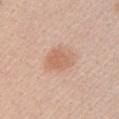Q: Was this lesion biopsied?
A: total-body-photography surveillance lesion; no biopsy
Q: Lesion location?
A: the chest
Q: Lesion size?
A: about 4 mm
Q: What is the imaging modality?
A: ~15 mm tile from a whole-body skin photo
Q: How was the tile lit?
A: white-light illumination
Q: Patient demographics?
A: female, approximately 40 years of age
Q: What did automated image analysis measure?
A: a mean CIELAB color near L≈63 a*≈21 b*≈32 and a lesion-to-skin contrast of about 6 (normalized; higher = more distinct); border irregularity of about 2 on a 0–10 scale, a color-variation rating of about 3/10, and radial color variation of about 1; a classifier nevus-likeness of about 70/100 and lesion-presence confidence of about 100/100